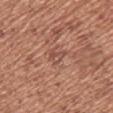Clinical impression: Part of a total-body skin-imaging series; this lesion was reviewed on a skin check and was not flagged for biopsy. Acquisition and patient details: Automated tile analysis of the lesion measured an average lesion color of about L≈50 a*≈23 b*≈27 (CIELAB), about 7 CIELAB-L* units darker than the surrounding skin, and a normalized lesion–skin contrast near 5.5. And it measured a classifier nevus-likeness of about 0/100. From the left upper arm. A roughly 15 mm field-of-view crop from a total-body skin photograph. A female patient, approximately 50 years of age.